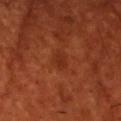Q: Was this lesion biopsied?
A: total-body-photography surveillance lesion; no biopsy
Q: Automated lesion metrics?
A: an area of roughly 3.5 mm², a shape eccentricity near 0.8, and a symmetry-axis asymmetry near 0.15; an automated nevus-likeness rating near 0 out of 100 and a detector confidence of about 100 out of 100 that the crop contains a lesion
Q: How large is the lesion?
A: ~3 mm (longest diameter)
Q: Where on the body is the lesion?
A: the head or neck
Q: What lighting was used for the tile?
A: cross-polarized illumination
Q: What kind of image is this?
A: ~15 mm tile from a whole-body skin photo
Q: Who is the patient?
A: male, about 50 years old